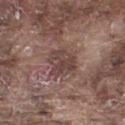Assessment:
Imaged during a routine full-body skin examination; the lesion was not biopsied and no histopathology is available.
Acquisition and patient details:
A male patient aged approximately 75. A 15 mm close-up extracted from a 3D total-body photography capture. The tile uses white-light illumination. Located on the leg. Measured at roughly 4 mm in maximum diameter. Automated tile analysis of the lesion measured a mean CIELAB color near L≈43 a*≈18 b*≈21 and a lesion-to-skin contrast of about 7 (normalized; higher = more distinct).